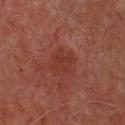  automated_metrics:
    cielab_L: 27
    cielab_a: 22
    cielab_b: 23
    border_irregularity_0_10: 3.0
    peripheral_color_asymmetry: 1.0
    nevus_likeness_0_100: 0
    lesion_detection_confidence_0_100: 100
  lighting: cross-polarized
  image:
    source: total-body photography crop
    field_of_view_mm: 15
  lesion_size:
    long_diameter_mm_approx: 3.0
  patient:
    sex: male
    age_approx: 65
  site: upper back